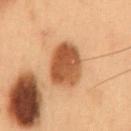Assessment: Recorded during total-body skin imaging; not selected for excision or biopsy. Image and clinical context: A male subject in their mid- to late 50s. Located on the back. Captured under cross-polarized illumination. Cropped from a total-body skin-imaging series; the visible field is about 15 mm.